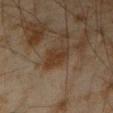| field | value |
|---|---|
| follow-up | no biopsy performed (imaged during a skin exam) |
| size | ~3.5 mm (longest diameter) |
| illumination | cross-polarized illumination |
| patient | male, approximately 45 years of age |
| imaging modality | total-body-photography crop, ~15 mm field of view |
| site | the left forearm |